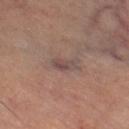This lesion was catalogued during total-body skin photography and was not selected for biopsy.
Approximately 3 mm at its widest.
A male patient aged around 65.
Imaged with cross-polarized lighting.
An algorithmic analysis of the crop reported a footprint of about 4 mm², a shape eccentricity near 0.85, and a shape-asymmetry score of about 0.2 (0 = symmetric). The analysis additionally found a mean CIELAB color near L≈46 a*≈16 b*≈19, a lesion–skin lightness drop of about 7, and a normalized lesion–skin contrast near 6.5. The analysis additionally found lesion-presence confidence of about 55/100.
The lesion is located on the leg.
Cropped from a total-body skin-imaging series; the visible field is about 15 mm.